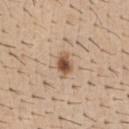Recorded during total-body skin imaging; not selected for excision or biopsy. This is a white-light tile. Approximately 3 mm at its widest. A male subject, roughly 40 years of age. Automated tile analysis of the lesion measured a footprint of about 5 mm² and an eccentricity of roughly 0.75. The analysis additionally found a lesion color around L≈54 a*≈18 b*≈31 in CIELAB, roughly 14 lightness units darker than nearby skin, and a lesion-to-skin contrast of about 9.5 (normalized; higher = more distinct). It also reported a border-irregularity index near 3/10 and a color-variation rating of about 4.5/10. A close-up tile cropped from a whole-body skin photograph, about 15 mm across. On the abdomen.